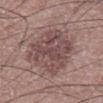Clinical impression: No biopsy was performed on this lesion — it was imaged during a full skin examination and was not determined to be concerning. Context: A lesion tile, about 15 mm wide, cut from a 3D total-body photograph. A male subject aged 58 to 62. On the abdomen. The recorded lesion diameter is about 7 mm. Automated image analysis of the tile measured a lesion area of about 31 mm², an eccentricity of roughly 0.65, and a symmetry-axis asymmetry near 0.15. And it measured a lesion–skin lightness drop of about 10 and a normalized border contrast of about 7.5. And it measured a border-irregularity index near 2.5/10, internal color variation of about 4.5 on a 0–10 scale, and radial color variation of about 1.5. The software also gave a classifier nevus-likeness of about 5/100 and a detector confidence of about 100 out of 100 that the crop contains a lesion.